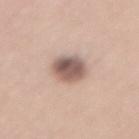biopsy_status: not biopsied; imaged during a skin examination
patient:
  sex: male
  age_approx: 60
lighting: white-light
site: lower back
image:
  source: total-body photography crop
  field_of_view_mm: 15